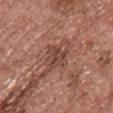The lesion was tiled from a total-body skin photograph and was not biopsied.
The total-body-photography lesion software estimated a mean CIELAB color near L≈44 a*≈22 b*≈27, a lesion–skin lightness drop of about 8, and a normalized lesion–skin contrast near 6.5. And it measured a border-irregularity index near 3.5/10, internal color variation of about 3.5 on a 0–10 scale, and a peripheral color-asymmetry measure near 1.5. It also reported an automated nevus-likeness rating near 0 out of 100 and a lesion-detection confidence of about 95/100.
The recorded lesion diameter is about 3 mm.
This image is a 15 mm lesion crop taken from a total-body photograph.
From the front of the torso.
Captured under white-light illumination.
The patient is a female approximately 65 years of age.